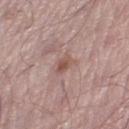| feature | finding |
|---|---|
| notes | catalogued during a skin exam; not biopsied |
| patient | male, roughly 55 years of age |
| lighting | white-light |
| diameter | ~2.5 mm (longest diameter) |
| body site | the left lower leg |
| image-analysis metrics | a footprint of about 3 mm² and a symmetry-axis asymmetry near 0.25; an automated nevus-likeness rating near 5 out of 100 and a lesion-detection confidence of about 100/100 |
| imaging modality | ~15 mm crop, total-body skin-cancer survey |